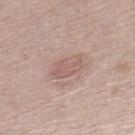| field | value |
|---|---|
| workup | catalogued during a skin exam; not biopsied |
| site | the right lower leg |
| subject | male, aged 63–67 |
| image | 15 mm crop, total-body photography |